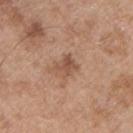Notes:
• follow-up: total-body-photography surveillance lesion; no biopsy
• TBP lesion metrics: an eccentricity of roughly 0.55 and a shape-asymmetry score of about 0.25 (0 = symmetric)
• patient: male, in their mid- to late 50s
• body site: the chest
• image: total-body-photography crop, ~15 mm field of view
• illumination: white-light illumination